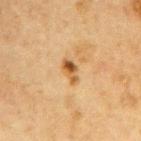No biopsy was performed on this lesion — it was imaged during a full skin examination and was not determined to be concerning.